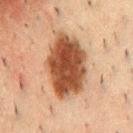| key | value |
|---|---|
| notes | total-body-photography surveillance lesion; no biopsy |
| body site | the chest |
| illumination | cross-polarized |
| image | total-body-photography crop, ~15 mm field of view |
| subject | male, aged approximately 50 |
| diameter | ~8 mm (longest diameter) |